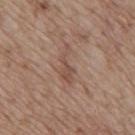notes=imaged on a skin check; not biopsied
tile lighting=white-light
site=the mid back
lesion size=~4.5 mm (longest diameter)
subject=male, about 70 years old
image source=~15 mm crop, total-body skin-cancer survey